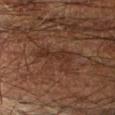Part of a total-body skin-imaging series; this lesion was reviewed on a skin check and was not flagged for biopsy.
This image is a 15 mm lesion crop taken from a total-body photograph.
A male patient aged 63–67.
The lesion is located on the right forearm.